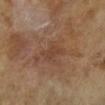Q: Was a biopsy performed?
A: imaged on a skin check; not biopsied
Q: How was this image acquired?
A: ~15 mm crop, total-body skin-cancer survey
Q: Lesion location?
A: the leg
Q: How was the tile lit?
A: cross-polarized illumination
Q: Who is the patient?
A: male, approximately 65 years of age